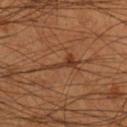Recorded during total-body skin imaging; not selected for excision or biopsy.
A close-up tile cropped from a whole-body skin photograph, about 15 mm across.
Located on the right lower leg.
A male subject, approximately 55 years of age.
The recorded lesion diameter is about 4 mm.
The total-body-photography lesion software estimated a lesion color around L≈34 a*≈21 b*≈29 in CIELAB, about 8 CIELAB-L* units darker than the surrounding skin, and a normalized lesion–skin contrast near 7.5. It also reported a border-irregularity rating of about 9/10.